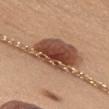<lesion>
<biopsy_status>not biopsied; imaged during a skin examination</biopsy_status>
<image>
  <source>total-body photography crop</source>
  <field_of_view_mm>15</field_of_view_mm>
</image>
<lesion_size>
  <long_diameter_mm_approx>8.0</long_diameter_mm_approx>
</lesion_size>
<patient>
  <sex>female</sex>
  <age_approx>40</age_approx>
</patient>
<lighting>white-light</lighting>
<site>front of the torso</site>
</lesion>